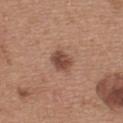follow-up: catalogued during a skin exam; not biopsied
patient: female, aged 33–37
location: the upper back
imaging modality: ~15 mm tile from a whole-body skin photo